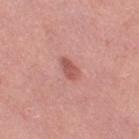Impression: This lesion was catalogued during total-body skin photography and was not selected for biopsy. Acquisition and patient details: Measured at roughly 3 mm in maximum diameter. A region of skin cropped from a whole-body photographic capture, roughly 15 mm wide. The lesion is on the right lower leg. Captured under white-light illumination. A female subject, in their 40s.